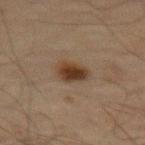{
  "site": "left thigh",
  "image": {
    "source": "total-body photography crop",
    "field_of_view_mm": 15
  },
  "lesion_size": {
    "long_diameter_mm_approx": 3.0
  },
  "patient": {
    "sex": "male",
    "age_approx": 70
  },
  "lighting": "cross-polarized"
}